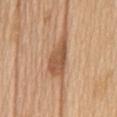Clinical impression:
Recorded during total-body skin imaging; not selected for excision or biopsy.
Background:
A region of skin cropped from a whole-body photographic capture, roughly 15 mm wide. A female patient about 70 years old. From the mid back.